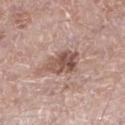The lesion was photographed on a routine skin check and not biopsied; there is no pathology result. This image is a 15 mm lesion crop taken from a total-body photograph. Imaged with white-light lighting. From the left lower leg. A male patient aged 48 to 52.